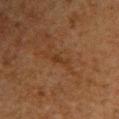notes: total-body-photography surveillance lesion; no biopsy
patient: female, aged around 60
lesion diameter: ≈2.5 mm
image: ~15 mm crop, total-body skin-cancer survey
illumination: cross-polarized illumination
location: the upper back